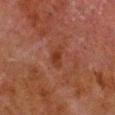| feature | finding |
|---|---|
| notes | catalogued during a skin exam; not biopsied |
| patient | male, about 80 years old |
| body site | the right lower leg |
| illumination | cross-polarized |
| acquisition | ~15 mm tile from a whole-body skin photo |
| diameter | ~2.5 mm (longest diameter) |